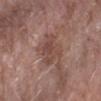- follow-up — no biopsy performed (imaged during a skin exam)
- lighting — white-light illumination
- lesion size — ≈4 mm
- location — the left forearm
- automated metrics — a mean CIELAB color near L≈46 a*≈19 b*≈23, a lesion–skin lightness drop of about 7, and a normalized border contrast of about 5.5; a classifier nevus-likeness of about 0/100
- subject — female, in their mid- to late 60s
- acquisition — ~15 mm crop, total-body skin-cancer survey